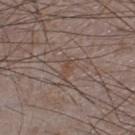notes: catalogued during a skin exam; not biopsied | lighting: white-light illumination | subject: male, aged approximately 75 | body site: the right lower leg | automated lesion analysis: an area of roughly 3.5 mm² and an outline eccentricity of about 0.9 (0 = round, 1 = elongated); a border-irregularity rating of about 3.5/10, a within-lesion color-variation index near 1.5/10, and a peripheral color-asymmetry measure near 0; a classifier nevus-likeness of about 0/100 and lesion-presence confidence of about 65/100 | imaging modality: ~15 mm tile from a whole-body skin photo.